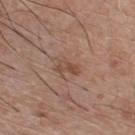notes = no biopsy performed (imaged during a skin exam); acquisition = 15 mm crop, total-body photography; lesion diameter = ~3 mm (longest diameter); subject = male, approximately 75 years of age; illumination = white-light illumination; site = the chest.